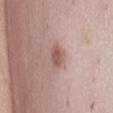| feature | finding |
|---|---|
| image | ~15 mm crop, total-body skin-cancer survey |
| anatomic site | the abdomen |
| patient | female, in their 40s |